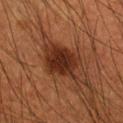Q: Was this lesion biopsied?
A: imaged on a skin check; not biopsied
Q: Automated lesion metrics?
A: a lesion area of about 13 mm², an eccentricity of roughly 0.65, and a symmetry-axis asymmetry near 0.25; about 11 CIELAB-L* units darker than the surrounding skin and a lesion-to-skin contrast of about 10.5 (normalized; higher = more distinct); border irregularity of about 3 on a 0–10 scale, internal color variation of about 3.5 on a 0–10 scale, and a peripheral color-asymmetry measure near 1; a lesion-detection confidence of about 100/100
Q: What is the lesion's diameter?
A: about 5 mm
Q: What are the patient's age and sex?
A: male, approximately 55 years of age
Q: What is the imaging modality?
A: ~15 mm tile from a whole-body skin photo
Q: What lighting was used for the tile?
A: cross-polarized illumination
Q: What is the anatomic site?
A: the left forearm